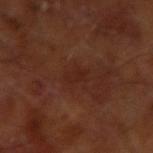| feature | finding |
|---|---|
| notes | imaged on a skin check; not biopsied |
| patient | male, roughly 65 years of age |
| anatomic site | the right upper arm |
| image source | ~15 mm crop, total-body skin-cancer survey |